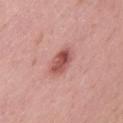The lesion was photographed on a routine skin check and not biopsied; there is no pathology result.